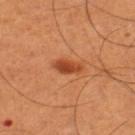Imaged with cross-polarized lighting. From the left thigh. A male patient about 50 years old. A region of skin cropped from a whole-body photographic capture, roughly 15 mm wide.